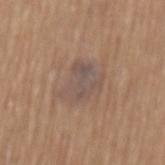Impression: Captured during whole-body skin photography for melanoma surveillance; the lesion was not biopsied. Image and clinical context: The lesion is located on the mid back. A 15 mm close-up tile from a total-body photography series done for melanoma screening. The patient is a male in their mid-60s. The recorded lesion diameter is about 4 mm.